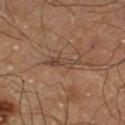Impression:
This lesion was catalogued during total-body skin photography and was not selected for biopsy.
Clinical summary:
A region of skin cropped from a whole-body photographic capture, roughly 15 mm wide. A male patient, aged around 60. On the left thigh.